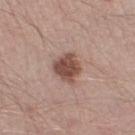This lesion was catalogued during total-body skin photography and was not selected for biopsy.
The lesion is located on the right lower leg.
Approximately 3.5 mm at its widest.
A male subject aged around 40.
Captured under white-light illumination.
Cropped from a total-body skin-imaging series; the visible field is about 15 mm.